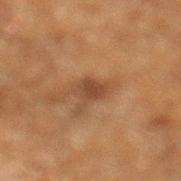image source = ~15 mm tile from a whole-body skin photo | size = ~2.5 mm (longest diameter) | patient = male, aged 58–62 | body site = the leg | lighting = cross-polarized illumination | automated metrics = a classifier nevus-likeness of about 15/100 and a lesion-detection confidence of about 100/100.